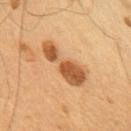<record>
<patient>
  <sex>male</sex>
  <age_approx>55</age_approx>
</patient>
<image>
  <source>total-body photography crop</source>
  <field_of_view_mm>15</field_of_view_mm>
</image>
<lighting>cross-polarized</lighting>
<lesion_size>
  <long_diameter_mm_approx>6.0</long_diameter_mm_approx>
</lesion_size>
<site>chest</site>
</record>